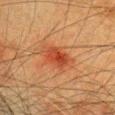Recorded during total-body skin imaging; not selected for excision or biopsy. Cropped from a whole-body photographic skin survey; the tile spans about 15 mm. On the head or neck. A female subject, in their mid-50s. Approximately 3.5 mm at its widest. This is a cross-polarized tile.